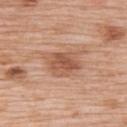| feature | finding |
|---|---|
| biopsy status | no biopsy performed (imaged during a skin exam) |
| subject | female, aged around 70 |
| automated metrics | a lesion area of about 11 mm², an eccentricity of roughly 0.7, and a symmetry-axis asymmetry near 0.25; a classifier nevus-likeness of about 65/100 |
| location | the back |
| lighting | white-light illumination |
| diameter | about 4.5 mm |
| image | ~15 mm tile from a whole-body skin photo |